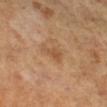* notes — imaged on a skin check; not biopsied
* size — ≈3 mm
* illumination — cross-polarized
* anatomic site — the right lower leg
* imaging modality — 15 mm crop, total-body photography
* patient — female, aged 53–57